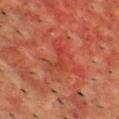  biopsy_status: not biopsied; imaged during a skin examination
  lesion_size:
    long_diameter_mm_approx: 3.0
  patient:
    sex: male
    age_approx: 55
  site: back
  lighting: cross-polarized
  image:
    source: total-body photography crop
    field_of_view_mm: 15